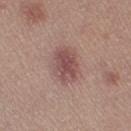Q: Is there a histopathology result?
A: catalogued during a skin exam; not biopsied
Q: What did automated image analysis measure?
A: a lesion area of about 12 mm², an outline eccentricity of about 0.75 (0 = round, 1 = elongated), and two-axis asymmetry of about 0.15; a lesion color around L≈50 a*≈21 b*≈21 in CIELAB, a lesion–skin lightness drop of about 10, and a lesion-to-skin contrast of about 7.5 (normalized; higher = more distinct)
Q: How large is the lesion?
A: ~5 mm (longest diameter)
Q: What kind of image is this?
A: 15 mm crop, total-body photography
Q: Patient demographics?
A: female, aged 18–22
Q: What is the anatomic site?
A: the leg